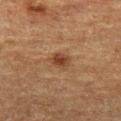| key | value |
|---|---|
| patient | male, in their mid- to late 70s |
| image | ~15 mm tile from a whole-body skin photo |
| location | the leg |
| lighting | cross-polarized |